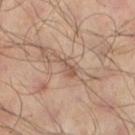biopsy status = no biopsy performed (imaged during a skin exam) | diameter = ~3 mm (longest diameter) | tile lighting = cross-polarized | location = the left lower leg | image = 15 mm crop, total-body photography | patient = male, approximately 65 years of age | TBP lesion metrics = a footprint of about 2.5 mm²; lesion-presence confidence of about 70/100.